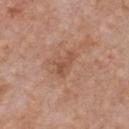Image and clinical context: The lesion's longest dimension is about 3 mm. A female patient aged around 40. Cropped from a whole-body photographic skin survey; the tile spans about 15 mm. Captured under white-light illumination. On the chest.